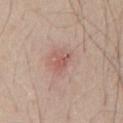Recorded during total-body skin imaging; not selected for excision or biopsy.
The lesion-visualizer software estimated a mean CIELAB color near L≈56 a*≈24 b*≈25 and a lesion–skin lightness drop of about 8. The software also gave lesion-presence confidence of about 100/100.
The lesion's longest dimension is about 2.5 mm.
A male patient in their mid-60s.
This image is a 15 mm lesion crop taken from a total-body photograph.
Located on the front of the torso.